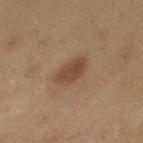<record>
<automated_metrics>
  <border_irregularity_0_10>2.0</border_irregularity_0_10>
  <color_variation_0_10>2.5</color_variation_0_10>
  <peripheral_color_asymmetry>1.0</peripheral_color_asymmetry>
</automated_metrics>
<image>
  <source>total-body photography crop</source>
  <field_of_view_mm>15</field_of_view_mm>
</image>
<patient>
  <sex>female</sex>
  <age_approx>60</age_approx>
</patient>
<site>left upper arm</site>
<lighting>cross-polarized</lighting>
</record>